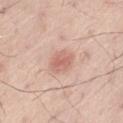| field | value |
|---|---|
| notes | total-body-photography surveillance lesion; no biopsy |
| TBP lesion metrics | a lesion area of about 6 mm², a shape eccentricity near 0.6, and a symmetry-axis asymmetry near 0.15; about 10 CIELAB-L* units darker than the surrounding skin and a normalized border contrast of about 6; border irregularity of about 1.5 on a 0–10 scale, internal color variation of about 2.5 on a 0–10 scale, and peripheral color asymmetry of about 0.5 |
| location | the left thigh |
| diameter | ~3 mm (longest diameter) |
| imaging modality | total-body-photography crop, ~15 mm field of view |
| patient | male, in their mid-50s |